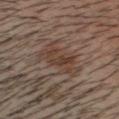Assessment:
No biopsy was performed on this lesion — it was imaged during a full skin examination and was not determined to be concerning.
Context:
A male patient, aged 38 to 42. A 15 mm crop from a total-body photograph taken for skin-cancer surveillance. From the head or neck.